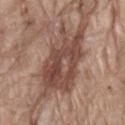This lesion was catalogued during total-body skin photography and was not selected for biopsy. Located on the lower back. The patient is a male approximately 80 years of age. A 15 mm close-up tile from a total-body photography series done for melanoma screening.